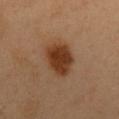biopsy_status: not biopsied; imaged during a skin examination
automated_metrics:
  area_mm2_approx: 14.0
  eccentricity: 0.75
  border_irregularity_0_10: 1.5
  color_variation_0_10: 4.0
  peripheral_color_asymmetry: 1.0
patient:
  sex: male
  age_approx: 50
site: back
image:
  source: total-body photography crop
  field_of_view_mm: 15
lighting: cross-polarized
lesion_size:
  long_diameter_mm_approx: 5.0